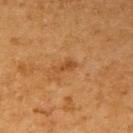* workup · imaged on a skin check; not biopsied
* image source · total-body-photography crop, ~15 mm field of view
* patient · male, aged 58 to 62
* image-analysis metrics · a mean CIELAB color near L≈48 a*≈25 b*≈41, roughly 8 lightness units darker than nearby skin, and a normalized lesion–skin contrast near 6.5; a detector confidence of about 100 out of 100 that the crop contains a lesion
* site · the right upper arm
* lighting · cross-polarized illumination